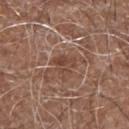Recorded during total-body skin imaging; not selected for excision or biopsy. A 15 mm close-up tile from a total-body photography series done for melanoma screening. The lesion is on the right upper arm. The patient is a male about 75 years old.